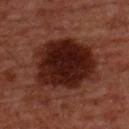{
  "biopsy_status": "not biopsied; imaged during a skin examination",
  "image": {
    "source": "total-body photography crop",
    "field_of_view_mm": 15
  },
  "patient": {
    "sex": "male",
    "age_approx": 60
  },
  "site": "chest"
}